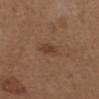| feature | finding |
|---|---|
| follow-up | total-body-photography surveillance lesion; no biopsy |
| acquisition | total-body-photography crop, ~15 mm field of view |
| subject | female, aged around 40 |
| lighting | white-light |
| location | the arm |
| size | ~3 mm (longest diameter) |